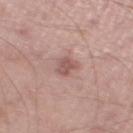Clinical impression: Imaged during a routine full-body skin examination; the lesion was not biopsied and no histopathology is available. Acquisition and patient details: The lesion-visualizer software estimated a lesion area of about 3.5 mm² and a shape-asymmetry score of about 0.3 (0 = symmetric). The software also gave a border-irregularity rating of about 2.5/10, internal color variation of about 2 on a 0–10 scale, and radial color variation of about 1. And it measured a classifier nevus-likeness of about 10/100 and lesion-presence confidence of about 100/100. Captured under white-light illumination. A male subject in their mid- to late 50s. The lesion's longest dimension is about 3 mm. A lesion tile, about 15 mm wide, cut from a 3D total-body photograph. The lesion is on the left lower leg.